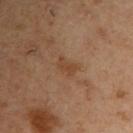Clinical impression: Imaged during a routine full-body skin examination; the lesion was not biopsied and no histopathology is available. Image and clinical context: From the right upper arm. This is a cross-polarized tile. About 2.5 mm across. A 15 mm close-up tile from a total-body photography series done for melanoma screening. The subject is a male aged approximately 55.